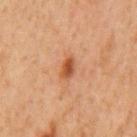The lesion was tiled from a total-body skin photograph and was not biopsied.
Automated image analysis of the tile measured an average lesion color of about L≈49 a*≈25 b*≈36 (CIELAB), a lesion–skin lightness drop of about 11, and a lesion-to-skin contrast of about 8.5 (normalized; higher = more distinct). The analysis additionally found a border-irregularity index near 2/10, a color-variation rating of about 3.5/10, and a peripheral color-asymmetry measure near 1. And it measured a nevus-likeness score of about 85/100 and a lesion-detection confidence of about 100/100.
Measured at roughly 2.5 mm in maximum diameter.
The patient is a male aged 58 to 62.
Cropped from a whole-body photographic skin survey; the tile spans about 15 mm.
The lesion is located on the mid back.
This is a cross-polarized tile.